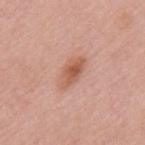| feature | finding |
|---|---|
| illumination | white-light |
| lesion size | about 4 mm |
| location | the mid back |
| patient | female, about 60 years old |
| image | total-body-photography crop, ~15 mm field of view |